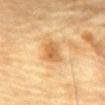The lesion was photographed on a routine skin check and not biopsied; there is no pathology result.
A 15 mm close-up tile from a total-body photography series done for melanoma screening.
Measured at roughly 3 mm in maximum diameter.
A male patient roughly 85 years of age or older.
The lesion is located on the abdomen.
Captured under cross-polarized illumination.